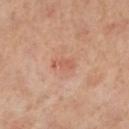Imaged during a routine full-body skin examination; the lesion was not biopsied and no histopathology is available. The subject is a female in their 60s. The recorded lesion diameter is about 3 mm. Located on the right leg. Imaged with cross-polarized lighting. A 15 mm close-up extracted from a 3D total-body photography capture.